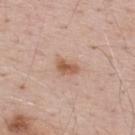Impression:
Recorded during total-body skin imaging; not selected for excision or biopsy.
Clinical summary:
The tile uses white-light illumination. A male subject, aged 68–72. A 15 mm close-up extracted from a 3D total-body photography capture. The lesion is located on the upper back. The total-body-photography lesion software estimated a footprint of about 4 mm², an eccentricity of roughly 0.8, and two-axis asymmetry of about 0.3. The analysis additionally found about 11 CIELAB-L* units darker than the surrounding skin and a lesion-to-skin contrast of about 8 (normalized; higher = more distinct).